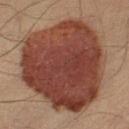Captured during whole-body skin photography for melanoma surveillance; the lesion was not biopsied. Longest diameter approximately 12 mm. Cropped from a whole-body photographic skin survey; the tile spans about 15 mm. From the left thigh. Imaged with cross-polarized lighting. The subject is a male about 40 years old. The lesion-visualizer software estimated an area of roughly 90 mm², an outline eccentricity of about 0.55 (0 = round, 1 = elongated), and a symmetry-axis asymmetry near 0.2. And it measured a lesion color around L≈36 a*≈21 b*≈24 in CIELAB and a normalized lesion–skin contrast near 12.5.